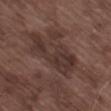biopsy status = imaged on a skin check; not biopsied
anatomic site = the left thigh
diameter = ≈8 mm
image = 15 mm crop, total-body photography
subject = male, about 75 years old
lighting = white-light illumination